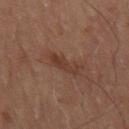biopsy status=no biopsy performed (imaged during a skin exam)
subject=male, about 70 years old
location=the right thigh
lesion size=about 3.5 mm
image source=~15 mm crop, total-body skin-cancer survey